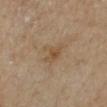Notes:
* follow-up · catalogued during a skin exam; not biopsied
* image · ~15 mm tile from a whole-body skin photo
* anatomic site · the leg
* patient · male, aged around 65
* TBP lesion metrics · an area of roughly 5 mm² and a shape-asymmetry score of about 0.35 (0 = symmetric); a lesion color around L≈50 a*≈15 b*≈32 in CIELAB and a lesion–skin lightness drop of about 7; a within-lesion color-variation index near 3/10 and a peripheral color-asymmetry measure near 1